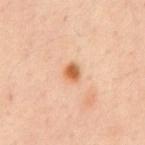Q: Was a biopsy performed?
A: imaged on a skin check; not biopsied
Q: What is the imaging modality?
A: ~15 mm tile from a whole-body skin photo
Q: Automated lesion metrics?
A: two-axis asymmetry of about 0.2; a mean CIELAB color near L≈60 a*≈25 b*≈39, about 14 CIELAB-L* units darker than the surrounding skin, and a normalized lesion–skin contrast near 10; border irregularity of about 1.5 on a 0–10 scale, internal color variation of about 3 on a 0–10 scale, and peripheral color asymmetry of about 1; an automated nevus-likeness rating near 95 out of 100 and a detector confidence of about 100 out of 100 that the crop contains a lesion
Q: How large is the lesion?
A: ≈2 mm
Q: Patient demographics?
A: male, about 65 years old
Q: What is the anatomic site?
A: the abdomen